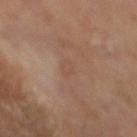This lesion was catalogued during total-body skin photography and was not selected for biopsy. The tile uses cross-polarized illumination. This image is a 15 mm lesion crop taken from a total-body photograph. The patient is a male roughly 70 years of age. The lesion-visualizer software estimated a mean CIELAB color near L≈48 a*≈18 b*≈27, about 4 CIELAB-L* units darker than the surrounding skin, and a normalized lesion–skin contrast near 3.5. It also reported a nevus-likeness score of about 0/100 and a lesion-detection confidence of about 100/100. On the mid back.